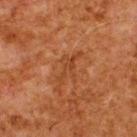biopsy status = total-body-photography surveillance lesion; no biopsy | subject = male, approximately 80 years of age | tile lighting = cross-polarized | acquisition = ~15 mm crop, total-body skin-cancer survey | location = the back.